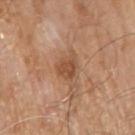Clinical impression: Part of a total-body skin-imaging series; this lesion was reviewed on a skin check and was not flagged for biopsy. Context: Cropped from a total-body skin-imaging series; the visible field is about 15 mm. The subject is a male roughly 80 years of age. The lesion is on the right upper arm.